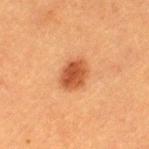Impression: No biopsy was performed on this lesion — it was imaged during a full skin examination and was not determined to be concerning. Clinical summary: Cropped from a total-body skin-imaging series; the visible field is about 15 mm. A female patient, approximately 40 years of age. An algorithmic analysis of the crop reported a shape-asymmetry score of about 0.15 (0 = symmetric). It also reported a lesion color around L≈44 a*≈25 b*≈35 in CIELAB, about 12 CIELAB-L* units darker than the surrounding skin, and a normalized lesion–skin contrast near 9. Located on the left thigh. The tile uses cross-polarized illumination. Approximately 3.5 mm at its widest.